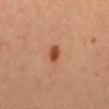biopsy status: catalogued during a skin exam; not biopsied
diameter: ~2.5 mm (longest diameter)
TBP lesion metrics: a lesion area of about 3.5 mm², an eccentricity of roughly 0.8, and a shape-asymmetry score of about 0.25 (0 = symmetric); a classifier nevus-likeness of about 100/100 and a detector confidence of about 100 out of 100 that the crop contains a lesion
anatomic site: the mid back
lighting: cross-polarized illumination
subject: female
image: ~15 mm crop, total-body skin-cancer survey